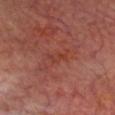Assessment: No biopsy was performed on this lesion — it was imaged during a full skin examination and was not determined to be concerning. Context: The lesion-visualizer software estimated an average lesion color of about L≈38 a*≈28 b*≈29 (CIELAB), about 4 CIELAB-L* units darker than the surrounding skin, and a normalized border contrast of about 5. It also reported a border-irregularity index near 4.5/10 and peripheral color asymmetry of about 0. The software also gave a classifier nevus-likeness of about 0/100 and lesion-presence confidence of about 100/100. Cropped from a total-body skin-imaging series; the visible field is about 15 mm. Imaged with cross-polarized lighting. Approximately 2.5 mm at its widest. The lesion is located on the head or neck. A male patient aged 63–67.